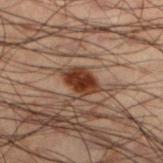Assessment:
Part of a total-body skin-imaging series; this lesion was reviewed on a skin check and was not flagged for biopsy.
Context:
The subject is a male in their 50s. This image is a 15 mm lesion crop taken from a total-body photograph. The recorded lesion diameter is about 3.5 mm. On the left lower leg.